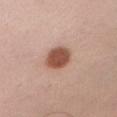{"biopsy_status": "not biopsied; imaged during a skin examination", "patient": {"sex": "male", "age_approx": 35}, "site": "left upper arm", "lesion_size": {"long_diameter_mm_approx": 3.5}, "image": {"source": "total-body photography crop", "field_of_view_mm": 15}, "lighting": "white-light"}